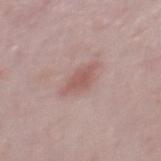{
  "biopsy_status": "not biopsied; imaged during a skin examination",
  "patient": {
    "sex": "male",
    "age_approx": 50
  },
  "image": {
    "source": "total-body photography crop",
    "field_of_view_mm": 15
  },
  "site": "mid back",
  "automated_metrics": {
    "border_irregularity_0_10": 2.5,
    "color_variation_0_10": 2.0,
    "peripheral_color_asymmetry": 0.5,
    "nevus_likeness_0_100": 20
  },
  "lighting": "white-light",
  "lesion_size": {
    "long_diameter_mm_approx": 4.0
  }
}